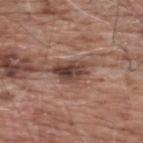Located on the upper back.
A 15 mm close-up tile from a total-body photography series done for melanoma screening.
The patient is a male aged around 60.